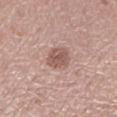Impression: No biopsy was performed on this lesion — it was imaged during a full skin examination and was not determined to be concerning. Clinical summary: The lesion's longest dimension is about 3.5 mm. This is a white-light tile. An algorithmic analysis of the crop reported a lesion area of about 6.5 mm² and a shape-asymmetry score of about 0.2 (0 = symmetric). The analysis additionally found a border-irregularity index near 2/10 and peripheral color asymmetry of about 1. The analysis additionally found lesion-presence confidence of about 100/100. A male subject, approximately 65 years of age. The lesion is on the left lower leg. Cropped from a whole-body photographic skin survey; the tile spans about 15 mm.